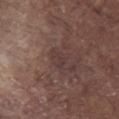{
  "biopsy_status": "not biopsied; imaged during a skin examination",
  "patient": {
    "sex": "male",
    "age_approx": 80
  },
  "image": {
    "source": "total-body photography crop",
    "field_of_view_mm": 15
  },
  "site": "chest",
  "lighting": "white-light"
}